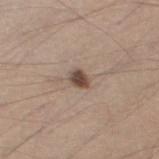Q: Is there a histopathology result?
A: total-body-photography surveillance lesion; no biopsy
Q: What is the lesion's diameter?
A: ~2 mm (longest diameter)
Q: What are the patient's age and sex?
A: male, aged approximately 40
Q: How was this image acquired?
A: ~15 mm tile from a whole-body skin photo
Q: Automated lesion metrics?
A: an outline eccentricity of about 0.65 (0 = round, 1 = elongated) and a symmetry-axis asymmetry near 0.25; a border-irregularity rating of about 2/10, internal color variation of about 3 on a 0–10 scale, and peripheral color asymmetry of about 1; an automated nevus-likeness rating near 95 out of 100
Q: What is the anatomic site?
A: the left thigh
Q: How was the tile lit?
A: white-light illumination